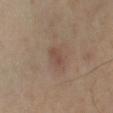Clinical impression: This lesion was catalogued during total-body skin photography and was not selected for biopsy. Background: About 2.5 mm across. A female subject, roughly 50 years of age. The tile uses cross-polarized illumination. A roughly 15 mm field-of-view crop from a total-body skin photograph. Located on the left lower leg.